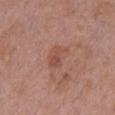<tbp_lesion>
<biopsy_status>not biopsied; imaged during a skin examination</biopsy_status>
<image>
  <source>total-body photography crop</source>
  <field_of_view_mm>15</field_of_view_mm>
</image>
<site>chest</site>
<patient>
  <sex>female</sex>
  <age_approx>50</age_approx>
</patient>
<automated_metrics>
  <border_irregularity_0_10>3.5</border_irregularity_0_10>
  <color_variation_0_10>2.5</color_variation_0_10>
  <nevus_likeness_0_100>0</nevus_likeness_0_100>
  <lesion_detection_confidence_0_100>100</lesion_detection_confidence_0_100>
</automated_metrics>
<lighting>white-light</lighting>
<lesion_size>
  <long_diameter_mm_approx>3.5</long_diameter_mm_approx>
</lesion_size>
</tbp_lesion>